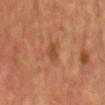Case summary:
• notes · catalogued during a skin exam; not biopsied
• acquisition · 15 mm crop, total-body photography
• automated metrics · border irregularity of about 3 on a 0–10 scale
• patient · male, in their mid-50s
• anatomic site · the chest